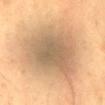notes = catalogued during a skin exam; not biopsied
patient = male, aged approximately 50
location = the abdomen
imaging modality = 15 mm crop, total-body photography
size = ≈9 mm